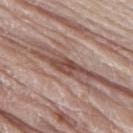Assessment:
Imaged during a routine full-body skin examination; the lesion was not biopsied and no histopathology is available.
Context:
Imaged with white-light lighting. A 15 mm close-up tile from a total-body photography series done for melanoma screening. Located on the leg. About 4 mm across. The patient is a male approximately 80 years of age.